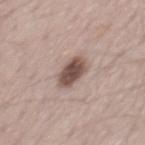The lesion was photographed on a routine skin check and not biopsied; there is no pathology result. From the mid back. This is a white-light tile. A male subject in their 70s. Longest diameter approximately 3.5 mm. A roughly 15 mm field-of-view crop from a total-body skin photograph.